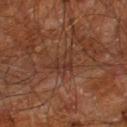follow-up: no biopsy performed (imaged during a skin exam) | patient: male, in their 60s | body site: the right leg | illumination: cross-polarized illumination | image source: ~15 mm tile from a whole-body skin photo | size: about 3.5 mm.